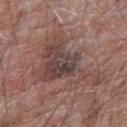Q: Is there a histopathology result?
A: total-body-photography surveillance lesion; no biopsy
Q: How was the tile lit?
A: white-light illumination
Q: Patient demographics?
A: male, aged 63 to 67
Q: What is the imaging modality?
A: total-body-photography crop, ~15 mm field of view
Q: Where on the body is the lesion?
A: the right upper arm